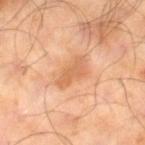Notes:
– follow-up — no biopsy performed (imaged during a skin exam)
– location — the leg
– tile lighting — cross-polarized illumination
– image source — total-body-photography crop, ~15 mm field of view
– patient — male, about 65 years old
– size — ~4 mm (longest diameter)